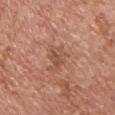No biopsy was performed on this lesion — it was imaged during a full skin examination and was not determined to be concerning.